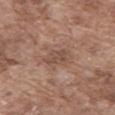This lesion was catalogued during total-body skin photography and was not selected for biopsy.
On the mid back.
A male patient, about 75 years old.
The recorded lesion diameter is about 2.5 mm.
A 15 mm close-up tile from a total-body photography series done for melanoma screening.